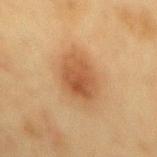notes=imaged on a skin check; not biopsied
image-analysis metrics=a lesion color around L≈46 a*≈20 b*≈33 in CIELAB, about 10 CIELAB-L* units darker than the surrounding skin, and a normalized lesion–skin contrast near 7.5; a border-irregularity rating of about 2/10, a color-variation rating of about 4/10, and peripheral color asymmetry of about 1.5
location=the mid back
imaging modality=15 mm crop, total-body photography
tile lighting=cross-polarized
diameter=≈5.5 mm
subject=female, aged 48 to 52